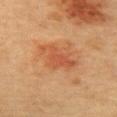Clinical impression: This lesion was catalogued during total-body skin photography and was not selected for biopsy. Acquisition and patient details: A 15 mm crop from a total-body photograph taken for skin-cancer surveillance. Approximately 5.5 mm at its widest. Captured under cross-polarized illumination. Located on the upper back. A female patient roughly 60 years of age.